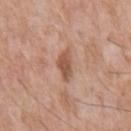Imaged during a routine full-body skin examination; the lesion was not biopsied and no histopathology is available.
A male patient approximately 60 years of age.
A 15 mm crop from a total-body photograph taken for skin-cancer surveillance.
The lesion is on the back.